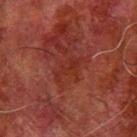Impression:
Recorded during total-body skin imaging; not selected for excision or biopsy.
Acquisition and patient details:
Approximately 3.5 mm at its widest. Located on the arm. An algorithmic analysis of the crop reported a mean CIELAB color near L≈24 a*≈25 b*≈24, about 4 CIELAB-L* units darker than the surrounding skin, and a normalized border contrast of about 5. The software also gave a border-irregularity rating of about 6/10, a within-lesion color-variation index near 1/10, and peripheral color asymmetry of about 0. Cropped from a total-body skin-imaging series; the visible field is about 15 mm. A male subject, aged 78 to 82. Imaged with cross-polarized lighting.